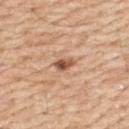| field | value |
|---|---|
| site | the upper back |
| illumination | white-light illumination |
| image source | total-body-photography crop, ~15 mm field of view |
| patient | male, aged approximately 60 |
| diameter | ~2.5 mm (longest diameter) |